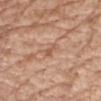Background: A female patient, about 75 years old. A roughly 15 mm field-of-view crop from a total-body skin photograph. Approximately 2.5 mm at its widest. The tile uses white-light illumination. The lesion is on the right upper arm.